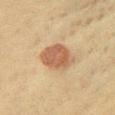Impression: No biopsy was performed on this lesion — it was imaged during a full skin examination and was not determined to be concerning. Clinical summary: Longest diameter approximately 4.5 mm. This image is a 15 mm lesion crop taken from a total-body photograph. Imaged with cross-polarized lighting. Located on the front of the torso. A female patient, aged approximately 40.